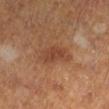Impression:
Recorded during total-body skin imaging; not selected for excision or biopsy.
Clinical summary:
Captured under cross-polarized illumination. The lesion is located on the left lower leg. A 15 mm close-up extracted from a 3D total-body photography capture. About 4 mm across. A male subject, aged approximately 60.